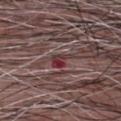Impression: This lesion was catalogued during total-body skin photography and was not selected for biopsy. Context: The lesion is on the chest. Approximately 2.5 mm at its widest. A 15 mm crop from a total-body photograph taken for skin-cancer surveillance. A male subject aged around 55.